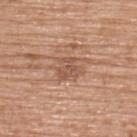Part of a total-body skin-imaging series; this lesion was reviewed on a skin check and was not flagged for biopsy. This image is a 15 mm lesion crop taken from a total-body photograph. Imaged with white-light lighting. From the upper back. The recorded lesion diameter is about 3 mm. A female patient, aged 63 to 67.